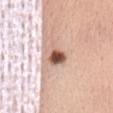Impression: Recorded during total-body skin imaging; not selected for excision or biopsy. Background: A 15 mm close-up extracted from a 3D total-body photography capture. The lesion is located on the front of the torso. A female patient, roughly 55 years of age.